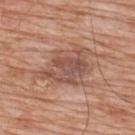The lesion was tiled from a total-body skin photograph and was not biopsied. On the back. Cropped from a total-body skin-imaging series; the visible field is about 15 mm. The subject is a male aged 78–82.